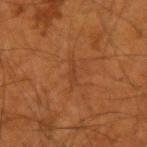Clinical impression:
Captured during whole-body skin photography for melanoma surveillance; the lesion was not biopsied.
Image and clinical context:
Captured under cross-polarized illumination. The subject is a male aged 53–57. The lesion is located on the right upper arm. A close-up tile cropped from a whole-body skin photograph, about 15 mm across.